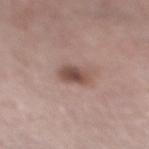The lesion was photographed on a routine skin check and not biopsied; there is no pathology result.
This image is a 15 mm lesion crop taken from a total-body photograph.
From the right lower leg.
The lesion-visualizer software estimated a lesion area of about 6 mm², a shape eccentricity near 0.45, and a symmetry-axis asymmetry near 0.2.
A female patient aged approximately 65.
The lesion's longest dimension is about 2.5 mm.
This is a white-light tile.